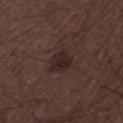Clinical impression:
The lesion was tiled from a total-body skin photograph and was not biopsied.
Image and clinical context:
Automated image analysis of the tile measured an area of roughly 5 mm², a shape eccentricity near 0.35, and a shape-asymmetry score of about 0.25 (0 = symmetric). The software also gave a border-irregularity index near 2.5/10, internal color variation of about 3 on a 0–10 scale, and a peripheral color-asymmetry measure near 1. It also reported a lesion-detection confidence of about 100/100. The lesion is located on the right thigh. A male subject roughly 50 years of age. A 15 mm close-up tile from a total-body photography series done for melanoma screening. Imaged with white-light lighting.